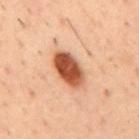The lesion was photographed on a routine skin check and not biopsied; there is no pathology result. An algorithmic analysis of the crop reported a border-irregularity index near 1.5/10, a color-variation rating of about 6/10, and peripheral color asymmetry of about 2. Measured at roughly 5 mm in maximum diameter. A male patient approximately 55 years of age. Captured under cross-polarized illumination. Cropped from a total-body skin-imaging series; the visible field is about 15 mm. On the mid back.